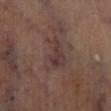The lesion was photographed on a routine skin check and not biopsied; there is no pathology result. The lesion is on the right lower leg. About 5 mm across. A 15 mm crop from a total-body photograph taken for skin-cancer surveillance. This is a cross-polarized tile. Automated tile analysis of the lesion measured a shape eccentricity near 0.8 and a symmetry-axis asymmetry near 0.45. The analysis additionally found internal color variation of about 4.5 on a 0–10 scale and a peripheral color-asymmetry measure near 1.5. The software also gave a nevus-likeness score of about 0/100 and lesion-presence confidence of about 70/100.